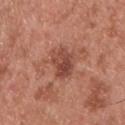This lesion was catalogued during total-body skin photography and was not selected for biopsy.
Measured at roughly 4 mm in maximum diameter.
The tile uses white-light illumination.
On the upper back.
The lesion-visualizer software estimated a lesion area of about 9 mm² and a shape eccentricity near 0.75. It also reported a nevus-likeness score of about 0/100.
The subject is a male approximately 55 years of age.
Cropped from a whole-body photographic skin survey; the tile spans about 15 mm.